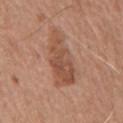Clinical impression:
The lesion was photographed on a routine skin check and not biopsied; there is no pathology result.
Image and clinical context:
Approximately 7 mm at its widest. From the chest. This is a white-light tile. Cropped from a total-body skin-imaging series; the visible field is about 15 mm. Automated image analysis of the tile measured a mean CIELAB color near L≈51 a*≈22 b*≈30, about 9 CIELAB-L* units darker than the surrounding skin, and a normalized border contrast of about 7. A male patient roughly 65 years of age.